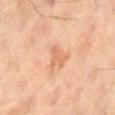Recorded during total-body skin imaging; not selected for excision or biopsy. Cropped from a total-body skin-imaging series; the visible field is about 15 mm. Longest diameter approximately 3 mm. Located on the right lower leg. A male subject, aged around 65. The total-body-photography lesion software estimated an area of roughly 4 mm², a shape eccentricity near 0.6, and a shape-asymmetry score of about 0.4 (0 = symmetric). The software also gave a detector confidence of about 100 out of 100 that the crop contains a lesion.